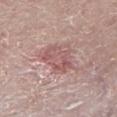| key | value |
|---|---|
| workup | no biopsy performed (imaged during a skin exam) |
| location | the leg |
| automated lesion analysis | an average lesion color of about L≈56 a*≈22 b*≈20 (CIELAB), about 9 CIELAB-L* units darker than the surrounding skin, and a normalized lesion–skin contrast near 6; a border-irregularity index near 3.5/10 |
| patient | male, in their mid- to late 60s |
| lesion size | ≈5 mm |
| lighting | white-light illumination |
| image | ~15 mm tile from a whole-body skin photo |